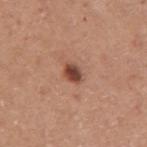workup: no biopsy performed (imaged during a skin exam); imaging modality: ~15 mm tile from a whole-body skin photo; location: the right upper arm; patient: female, approximately 55 years of age.